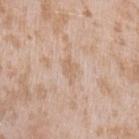Clinical summary:
Measured at roughly 2.5 mm in maximum diameter. Cropped from a whole-body photographic skin survey; the tile spans about 15 mm. The lesion is located on the left upper arm. A female patient in their mid-20s.